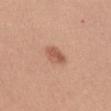Imaged during a routine full-body skin examination; the lesion was not biopsied and no histopathology is available.
About 2.5 mm across.
A female subject, approximately 35 years of age.
A 15 mm close-up extracted from a 3D total-body photography capture.
The lesion is on the abdomen.
Automated image analysis of the tile measured an automated nevus-likeness rating near 95 out of 100 and a detector confidence of about 100 out of 100 that the crop contains a lesion.